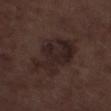follow-up = total-body-photography surveillance lesion; no biopsy | image source = ~15 mm crop, total-body skin-cancer survey | site = the left lower leg | patient = male, aged 68–72 | diameter = ~7.5 mm (longest diameter).